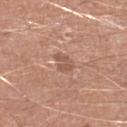This lesion was catalogued during total-body skin photography and was not selected for biopsy.
The lesion's longest dimension is about 2.5 mm.
A male subject, aged 78–82.
The lesion is located on the left lower leg.
A roughly 15 mm field-of-view crop from a total-body skin photograph.
An algorithmic analysis of the crop reported a footprint of about 3 mm², an outline eccentricity of about 0.8 (0 = round, 1 = elongated), and a symmetry-axis asymmetry near 0.45. It also reported a nevus-likeness score of about 0/100.
Captured under white-light illumination.